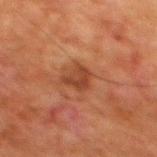biopsy_status: not biopsied; imaged during a skin examination
patient:
  sex: male
  age_approx: 80
site: mid back
lighting: cross-polarized
image:
  source: total-body photography crop
  field_of_view_mm: 15
automated_metrics:
  cielab_L: 33
  cielab_a: 22
  cielab_b: 28
  vs_skin_darker_L: 8.0
  vs_skin_contrast_norm: 7.0
  nevus_likeness_0_100: 0
  lesion_detection_confidence_0_100: 100
lesion_size:
  long_diameter_mm_approx: 3.0